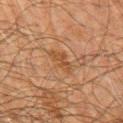diameter: about 4 mm | anatomic site: the chest | tile lighting: cross-polarized illumination | automated lesion analysis: a lesion color around L≈38 a*≈18 b*≈30 in CIELAB, a lesion–skin lightness drop of about 6, and a normalized lesion–skin contrast near 6.5; a border-irregularity index near 5/10, a color-variation rating of about 2/10, and peripheral color asymmetry of about 0.5 | subject: male, in their 60s | acquisition: 15 mm crop, total-body photography.